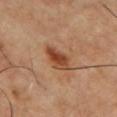Clinical impression: The lesion was photographed on a routine skin check and not biopsied; there is no pathology result. Image and clinical context: The lesion is located on the chest. A region of skin cropped from a whole-body photographic capture, roughly 15 mm wide. A male patient aged 53 to 57. This is a cross-polarized tile. The recorded lesion diameter is about 4 mm.